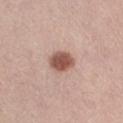follow-up: catalogued during a skin exam; not biopsied | imaging modality: total-body-photography crop, ~15 mm field of view | site: the right thigh | patient: female, in their 50s | image-analysis metrics: an area of roughly 7 mm², a shape eccentricity near 0.6, and a symmetry-axis asymmetry near 0.15; a lesion–skin lightness drop of about 15 and a normalized border contrast of about 10; a border-irregularity index near 1/10 and a peripheral color-asymmetry measure near 1; a nevus-likeness score of about 100/100 and a lesion-detection confidence of about 100/100.